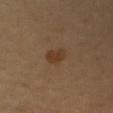Recorded during total-body skin imaging; not selected for excision or biopsy. This is a cross-polarized tile. A male patient, aged approximately 55. The total-body-photography lesion software estimated a border-irregularity rating of about 2.5/10, internal color variation of about 2 on a 0–10 scale, and a peripheral color-asymmetry measure near 1. Measured at roughly 2.5 mm in maximum diameter. The lesion is located on the right upper arm. A lesion tile, about 15 mm wide, cut from a 3D total-body photograph.